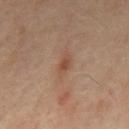Notes:
• follow-up · catalogued during a skin exam; not biopsied
• image · ~15 mm tile from a whole-body skin photo
• illumination · cross-polarized
• size · ~3 mm (longest diameter)
• site · the mid back
• patient · male, aged 63 to 67